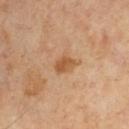| field | value |
|---|---|
| follow-up | catalogued during a skin exam; not biopsied |
| site | the chest |
| patient | male, about 65 years old |
| lighting | cross-polarized |
| diameter | ≈2.5 mm |
| image source | ~15 mm tile from a whole-body skin photo |
| automated lesion analysis | two-axis asymmetry of about 0.25; a nevus-likeness score of about 55/100 and lesion-presence confidence of about 100/100 |